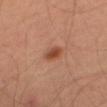  biopsy_status: not biopsied; imaged during a skin examination
  lesion_size:
    long_diameter_mm_approx: 3.0
  patient:
    sex: male
    age_approx: 65
  site: left thigh
  image:
    source: total-body photography crop
    field_of_view_mm: 15
  lighting: cross-polarized
  automated_metrics:
    area_mm2_approx: 4.0
    shape_asymmetry: 0.15
    border_irregularity_0_10: 1.5
    color_variation_0_10: 2.0
    peripheral_color_asymmetry: 0.5